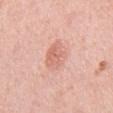Imaged with white-light lighting. This image is a 15 mm lesion crop taken from a total-body photograph. A male patient, about 55 years old. The lesion's longest dimension is about 4 mm. The lesion-visualizer software estimated a footprint of about 8 mm², an eccentricity of roughly 0.8, and a symmetry-axis asymmetry near 0.15. The lesion is located on the chest.